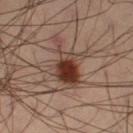The lesion was photographed on a routine skin check and not biopsied; there is no pathology result.
Located on the left thigh.
Longest diameter approximately 6 mm.
The lesion-visualizer software estimated an outline eccentricity of about 0.8 (0 = round, 1 = elongated). The analysis additionally found a lesion color around L≈38 a*≈19 b*≈25 in CIELAB, a lesion–skin lightness drop of about 13, and a lesion-to-skin contrast of about 11 (normalized; higher = more distinct).
A male subject, about 40 years old.
A 15 mm close-up extracted from a 3D total-body photography capture.
The tile uses cross-polarized illumination.